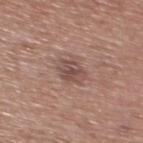Impression: This lesion was catalogued during total-body skin photography and was not selected for biopsy. Context: A male subject aged 43 to 47. The tile uses white-light illumination. Measured at roughly 3 mm in maximum diameter. The lesion is on the chest. Cropped from a whole-body photographic skin survey; the tile spans about 15 mm.